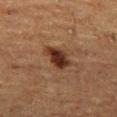Impression:
Part of a total-body skin-imaging series; this lesion was reviewed on a skin check and was not flagged for biopsy.
Clinical summary:
From the left thigh. A male subject roughly 75 years of age. Cropped from a whole-body photographic skin survey; the tile spans about 15 mm.